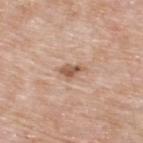<record>
<biopsy_status>not biopsied; imaged during a skin examination</biopsy_status>
<image>
  <source>total-body photography crop</source>
  <field_of_view_mm>15</field_of_view_mm>
</image>
<lesion_size>
  <long_diameter_mm_approx>2.5</long_diameter_mm_approx>
</lesion_size>
<lighting>white-light</lighting>
<patient>
  <sex>male</sex>
  <age_approx>60</age_approx>
</patient>
<automated_metrics>
  <cielab_L>57</cielab_L>
  <cielab_a>19</cielab_a>
  <cielab_b>30</cielab_b>
</automated_metrics>
<site>upper back</site>
</record>